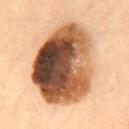| key | value |
|---|---|
| follow-up | catalogued during a skin exam; not biopsied |
| patient | female, roughly 55 years of age |
| lesion size | ~9.5 mm (longest diameter) |
| image source | total-body-photography crop, ~15 mm field of view |
| location | the mid back |